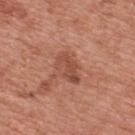Part of a total-body skin-imaging series; this lesion was reviewed on a skin check and was not flagged for biopsy.